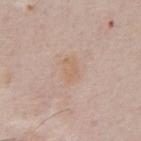Recorded during total-body skin imaging; not selected for excision or biopsy.
The subject is a male in their 50s.
A lesion tile, about 15 mm wide, cut from a 3D total-body photograph.
From the chest.